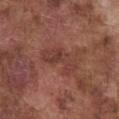{
  "biopsy_status": "not biopsied; imaged during a skin examination",
  "patient": {
    "sex": "male",
    "age_approx": 75
  },
  "image": {
    "source": "total-body photography crop",
    "field_of_view_mm": 15
  },
  "site": "chest"
}